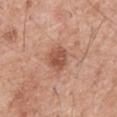Notes:
• workup · no biopsy performed (imaged during a skin exam)